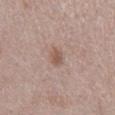Clinical impression: Captured during whole-body skin photography for melanoma surveillance; the lesion was not biopsied. Image and clinical context: Approximately 2.5 mm at its widest. The patient is a male in their 70s. Cropped from a whole-body photographic skin survey; the tile spans about 15 mm. The lesion is located on the back.